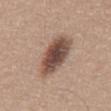* workup: no biopsy performed (imaged during a skin exam)
* imaging modality: ~15 mm tile from a whole-body skin photo
* size: ~5.5 mm (longest diameter)
* TBP lesion metrics: a mean CIELAB color near L≈48 a*≈17 b*≈25 and a normalized border contrast of about 11; a border-irregularity index near 2/10, internal color variation of about 6 on a 0–10 scale, and a peripheral color-asymmetry measure near 1.5; a classifier nevus-likeness of about 75/100 and lesion-presence confidence of about 100/100
* subject: male, in their mid- to late 60s
* location: the back